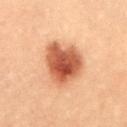Impression: Captured during whole-body skin photography for melanoma surveillance; the lesion was not biopsied. Clinical summary: The total-body-photography lesion software estimated a lesion area of about 18 mm², a shape eccentricity near 0.55, and a shape-asymmetry score of about 0.25 (0 = symmetric). The analysis additionally found an average lesion color of about L≈44 a*≈23 b*≈30 (CIELAB), a lesion–skin lightness drop of about 15, and a normalized lesion–skin contrast near 11. The patient is a female aged 18 to 22. Cropped from a whole-body photographic skin survey; the tile spans about 15 mm. Imaged with cross-polarized lighting. The recorded lesion diameter is about 5.5 mm. The lesion is on the abdomen.